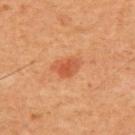This lesion was catalogued during total-body skin photography and was not selected for biopsy. This is a cross-polarized tile. Approximately 3 mm at its widest. The lesion-visualizer software estimated a footprint of about 5 mm², an eccentricity of roughly 0.7, and a symmetry-axis asymmetry near 0.35. The analysis additionally found an average lesion color of about L≈49 a*≈29 b*≈36 (CIELAB), about 9 CIELAB-L* units darker than the surrounding skin, and a lesion-to-skin contrast of about 6.5 (normalized; higher = more distinct). And it measured a classifier nevus-likeness of about 75/100 and a lesion-detection confidence of about 100/100. A male subject aged approximately 60. The lesion is located on the front of the torso. Cropped from a total-body skin-imaging series; the visible field is about 15 mm.